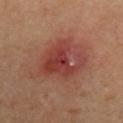notes = total-body-photography surveillance lesion; no biopsy | image = total-body-photography crop, ~15 mm field of view | patient = male, aged 63–67 | body site = the left upper arm | diameter = ≈7 mm.